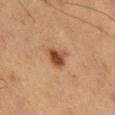Part of a total-body skin-imaging series; this lesion was reviewed on a skin check and was not flagged for biopsy.
Imaged with cross-polarized lighting.
The lesion is on the mid back.
A roughly 15 mm field-of-view crop from a total-body skin photograph.
The lesion's longest dimension is about 2.5 mm.
The patient is a male aged 58 to 62.